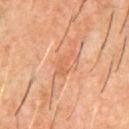Case summary:
– workup: imaged on a skin check; not biopsied
– image-analysis metrics: an automated nevus-likeness rating near 0 out of 100 and a detector confidence of about 75 out of 100 that the crop contains a lesion
– size: ~3.5 mm (longest diameter)
– patient: male, aged approximately 60
– acquisition: ~15 mm crop, total-body skin-cancer survey
– body site: the front of the torso